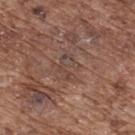<record>
<biopsy_status>not biopsied; imaged during a skin examination</biopsy_status>
<patient>
  <sex>male</sex>
  <age_approx>75</age_approx>
</patient>
<lighting>white-light</lighting>
<automated_metrics>
  <area_mm2_approx>6.0</area_mm2_approx>
  <eccentricity>0.7</eccentricity>
  <shape_asymmetry>0.45</shape_asymmetry>
  <cielab_L>44</cielab_L>
  <cielab_a>17</cielab_a>
  <cielab_b>23</cielab_b>
  <vs_skin_darker_L>5.0</vs_skin_darker_L>
  <vs_skin_contrast_norm>5.0</vs_skin_contrast_norm>
  <color_variation_0_10>5.5</color_variation_0_10>
  <nevus_likeness_0_100>0</nevus_likeness_0_100>
  <lesion_detection_confidence_0_100>55</lesion_detection_confidence_0_100>
</automated_metrics>
<lesion_size>
  <long_diameter_mm_approx>3.0</long_diameter_mm_approx>
</lesion_size>
<image>
  <source>total-body photography crop</source>
  <field_of_view_mm>15</field_of_view_mm>
</image>
<site>upper back</site>
</record>